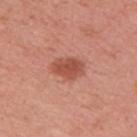Assessment: This lesion was catalogued during total-body skin photography and was not selected for biopsy. Context: A 15 mm close-up extracted from a 3D total-body photography capture. Located on the right upper arm. The patient is a female aged approximately 50.